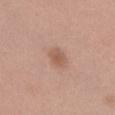Clinical impression: This lesion was catalogued during total-body skin photography and was not selected for biopsy. Clinical summary: Automated tile analysis of the lesion measured an average lesion color of about L≈57 a*≈20 b*≈28 (CIELAB) and roughly 8 lightness units darker than nearby skin. It also reported border irregularity of about 2 on a 0–10 scale, a color-variation rating of about 2/10, and peripheral color asymmetry of about 0.5. The software also gave a nevus-likeness score of about 65/100. Approximately 3 mm at its widest. This is a white-light tile. A female subject about 40 years old. On the abdomen. A region of skin cropped from a whole-body photographic capture, roughly 15 mm wide.